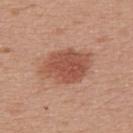{
  "biopsy_status": "not biopsied; imaged during a skin examination",
  "automated_metrics": {
    "nevus_likeness_0_100": 95,
    "lesion_detection_confidence_0_100": 100
  },
  "image": {
    "source": "total-body photography crop",
    "field_of_view_mm": 15
  },
  "patient": {
    "sex": "female",
    "age_approx": 70
  },
  "lesion_size": {
    "long_diameter_mm_approx": 6.5
  },
  "lighting": "white-light",
  "site": "upper back"
}